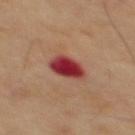  lesion_size:
    long_diameter_mm_approx: 3.5
  automated_metrics:
    nevus_likeness_0_100: 0
    lesion_detection_confidence_0_100: 100
  site: mid back
  patient:
    sex: male
    age_approx: 70
  image:
    source: total-body photography crop
    field_of_view_mm: 15